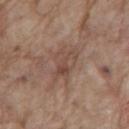The lesion was photographed on a routine skin check and not biopsied; there is no pathology result. A male subject roughly 70 years of age. Imaged with white-light lighting. A close-up tile cropped from a whole-body skin photograph, about 15 mm across. The lesion is on the mid back.